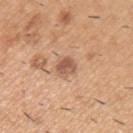follow-up: catalogued during a skin exam; not biopsied
subject: male, aged 38–42
tile lighting: white-light
size: ≈2.5 mm
location: the left upper arm
imaging modality: ~15 mm crop, total-body skin-cancer survey
automated metrics: roughly 12 lightness units darker than nearby skin; a border-irregularity rating of about 2/10, internal color variation of about 3 on a 0–10 scale, and radial color variation of about 1; an automated nevus-likeness rating near 45 out of 100 and lesion-presence confidence of about 100/100